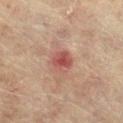  biopsy_status: not biopsied; imaged during a skin examination
  lighting: cross-polarized
  image:
    source: total-body photography crop
    field_of_view_mm: 15
  site: right lower leg
  patient:
    sex: female
    age_approx: 80
  lesion_size:
    long_diameter_mm_approx: 2.5